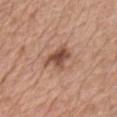Part of a total-body skin-imaging series; this lesion was reviewed on a skin check and was not flagged for biopsy.
A region of skin cropped from a whole-body photographic capture, roughly 15 mm wide.
Located on the chest.
Captured under white-light illumination.
A male patient, aged 58–62.
Longest diameter approximately 4 mm.